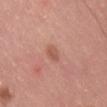  biopsy_status: not biopsied; imaged during a skin examination
  patient:
    sex: male
    age_approx: 40
  lesion_size:
    long_diameter_mm_approx: 3.0
  automated_metrics:
    area_mm2_approx: 3.0
    eccentricity: 0.9
    shape_asymmetry: 0.35
    cielab_L: 55
    cielab_a: 24
    cielab_b: 29
    vs_skin_darker_L: 8.0
    vs_skin_contrast_norm: 6.0
    border_irregularity_0_10: 3.5
  site: back
  lighting: white-light
  image:
    source: total-body photography crop
    field_of_view_mm: 15